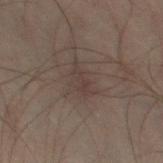The patient is a male aged approximately 50. Approximately 2.5 mm at its widest. This image is a 15 mm lesion crop taken from a total-body photograph. The lesion is on the left thigh.